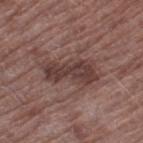Q: Is there a histopathology result?
A: catalogued during a skin exam; not biopsied
Q: What is the imaging modality?
A: total-body-photography crop, ~15 mm field of view
Q: Where on the body is the lesion?
A: the left thigh
Q: Lesion size?
A: ≈6.5 mm
Q: What are the patient's age and sex?
A: male, aged 68–72
Q: What did automated image analysis measure?
A: a footprint of about 15 mm², an outline eccentricity of about 0.9 (0 = round, 1 = elongated), and two-axis asymmetry of about 0.35; roughly 10 lightness units darker than nearby skin; a nevus-likeness score of about 0/100
Q: Illumination type?
A: white-light